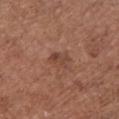anatomic site: the chest
illumination: white-light
imaging modality: ~15 mm tile from a whole-body skin photo
automated lesion analysis: an area of roughly 3.5 mm², an outline eccentricity of about 0.75 (0 = round, 1 = elongated), and a symmetry-axis asymmetry near 0.3; a border-irregularity index near 3.5/10, internal color variation of about 3 on a 0–10 scale, and radial color variation of about 1; a lesion-detection confidence of about 100/100
lesion diameter: about 2.5 mm
patient: female, about 75 years old